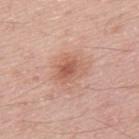This lesion was catalogued during total-body skin photography and was not selected for biopsy. Automated tile analysis of the lesion measured a nevus-likeness score of about 70/100. This is a white-light tile. A male patient, aged 48 to 52. The lesion is on the upper back. The lesion's longest dimension is about 3.5 mm. A roughly 15 mm field-of-view crop from a total-body skin photograph.